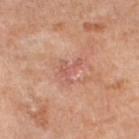• follow-up: catalogued during a skin exam; not biopsied
• image source: total-body-photography crop, ~15 mm field of view
• lesion diameter: ≈3 mm
• location: the left lower leg
• illumination: cross-polarized illumination
• automated lesion analysis: an outline eccentricity of about 0.7 (0 = round, 1 = elongated) and two-axis asymmetry of about 0.65; a lesion color around L≈56 a*≈25 b*≈27 in CIELAB, about 6 CIELAB-L* units darker than the surrounding skin, and a lesion-to-skin contrast of about 4.5 (normalized; higher = more distinct)
• patient: in their mid-50s